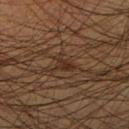This lesion was catalogued during total-body skin photography and was not selected for biopsy.
Located on the right lower leg.
A male patient in their mid- to late 40s.
Cropped from a whole-body photographic skin survey; the tile spans about 15 mm.
Imaged with cross-polarized lighting.